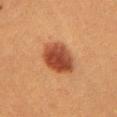Impression:
Captured during whole-body skin photography for melanoma surveillance; the lesion was not biopsied.
Image and clinical context:
The lesion is on the chest. A female patient aged 53–57. This image is a 15 mm lesion crop taken from a total-body photograph.